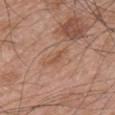notes=no biopsy performed (imaged during a skin exam)
subject=male, aged 68 to 72
imaging modality=~15 mm tile from a whole-body skin photo
body site=the chest